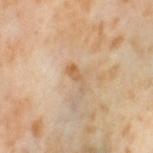Impression: The lesion was photographed on a routine skin check and not biopsied; there is no pathology result. Acquisition and patient details: A female subject, aged around 55. This is a cross-polarized tile. A 15 mm close-up tile from a total-body photography series done for melanoma screening. The lesion is located on the leg. The total-body-photography lesion software estimated an eccentricity of roughly 0.9. And it measured an automated nevus-likeness rating near 0 out of 100 and a detector confidence of about 100 out of 100 that the crop contains a lesion.